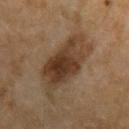Clinical impression: This lesion was catalogued during total-body skin photography and was not selected for biopsy. Background: About 7.5 mm across. A roughly 15 mm field-of-view crop from a total-body skin photograph. This is a cross-polarized tile. The lesion-visualizer software estimated a border-irregularity index near 3.5/10 and radial color variation of about 1.5. And it measured an automated nevus-likeness rating near 85 out of 100 and a detector confidence of about 100 out of 100 that the crop contains a lesion. The patient is a female approximately 60 years of age. From the left forearm.